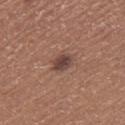| feature | finding |
|---|---|
| notes | catalogued during a skin exam; not biopsied |
| tile lighting | white-light illumination |
| lesion size | about 3 mm |
| patient | female, aged 33 to 37 |
| anatomic site | the right thigh |
| acquisition | total-body-photography crop, ~15 mm field of view |
| automated lesion analysis | a lesion area of about 4.5 mm², an eccentricity of roughly 0.65, and a shape-asymmetry score of about 0.15 (0 = symmetric); an automated nevus-likeness rating near 85 out of 100 |